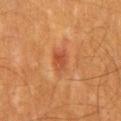The lesion was photographed on a routine skin check and not biopsied; there is no pathology result. A close-up tile cropped from a whole-body skin photograph, about 15 mm across. A male subject, aged around 55. The recorded lesion diameter is about 2.5 mm. Automated tile analysis of the lesion measured a lesion color around L≈48 a*≈31 b*≈38 in CIELAB, about 9 CIELAB-L* units darker than the surrounding skin, and a lesion-to-skin contrast of about 6.5 (normalized; higher = more distinct). It also reported border irregularity of about 1.5 on a 0–10 scale, a within-lesion color-variation index near 2/10, and radial color variation of about 0.5. It also reported a nevus-likeness score of about 55/100 and a lesion-detection confidence of about 100/100. The lesion is located on the mid back.